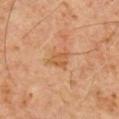Imaged during a routine full-body skin examination; the lesion was not biopsied and no histopathology is available.
About 3 mm across.
A male patient roughly 60 years of age.
Located on the chest.
A 15 mm close-up extracted from a 3D total-body photography capture.